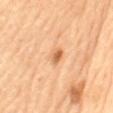subject: female, about 65 years old
location: the mid back
lesion size: ≈2 mm
automated lesion analysis: an eccentricity of roughly 0.75 and a shape-asymmetry score of about 0.15 (0 = symmetric); about 12 CIELAB-L* units darker than the surrounding skin and a normalized border contrast of about 7.5; internal color variation of about 2.5 on a 0–10 scale
lighting: cross-polarized
image: ~15 mm crop, total-body skin-cancer survey